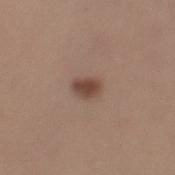• anatomic site — the left lower leg
• image — ~15 mm tile from a whole-body skin photo
• patient — female, aged 43–47